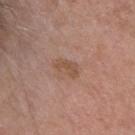The lesion was tiled from a total-body skin photograph and was not biopsied. On the head or neck. This is a white-light tile. The subject is a male aged 38–42. The lesion-visualizer software estimated an automated nevus-likeness rating near 0 out of 100 and a lesion-detection confidence of about 100/100. Cropped from a whole-body photographic skin survey; the tile spans about 15 mm. About 3 mm across.